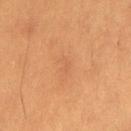The lesion was photographed on a routine skin check and not biopsied; there is no pathology result. A female subject approximately 40 years of age. The lesion's longest dimension is about 2 mm. Automated image analysis of the tile measured an eccentricity of roughly 0.85 and two-axis asymmetry of about 0.35. This image is a 15 mm lesion crop taken from a total-body photograph. The lesion is located on the left thigh. Captured under cross-polarized illumination.